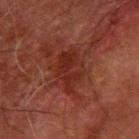Recorded during total-body skin imaging; not selected for excision or biopsy.
The lesion's longest dimension is about 5 mm.
Cropped from a total-body skin-imaging series; the visible field is about 15 mm.
Captured under cross-polarized illumination.
The lesion is located on the left forearm.
Automated image analysis of the tile measured a footprint of about 11 mm² and a shape-asymmetry score of about 0.25 (0 = symmetric). The software also gave an average lesion color of about L≈22 a*≈22 b*≈23 (CIELAB), roughly 6 lightness units darker than nearby skin, and a lesion-to-skin contrast of about 7 (normalized; higher = more distinct). It also reported a border-irregularity rating of about 2.5/10 and a color-variation rating of about 3.5/10. The analysis additionally found a nevus-likeness score of about 0/100 and a detector confidence of about 95 out of 100 that the crop contains a lesion.
The patient is a male aged 78 to 82.